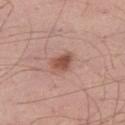Case summary:
* workup — no biopsy performed (imaged during a skin exam)
* tile lighting — white-light
* subject — male, aged approximately 30
* anatomic site — the right thigh
* diameter — ~3 mm (longest diameter)
* image — total-body-photography crop, ~15 mm field of view